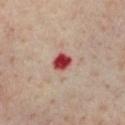No biopsy was performed on this lesion — it was imaged during a full skin examination and was not determined to be concerning.
This is a cross-polarized tile.
The subject is a male aged approximately 65.
The lesion is on the chest.
Longest diameter approximately 2.5 mm.
Automated tile analysis of the lesion measured a lesion area of about 4 mm², a shape eccentricity near 0.5, and two-axis asymmetry of about 0.15. The software also gave internal color variation of about 3 on a 0–10 scale and a peripheral color-asymmetry measure near 1. The software also gave a nevus-likeness score of about 0/100.
A 15 mm close-up extracted from a 3D total-body photography capture.